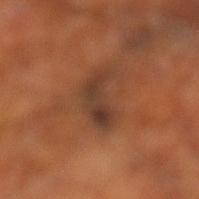workup — catalogued during a skin exam; not biopsied
image source — total-body-photography crop, ~15 mm field of view
automated metrics — an eccentricity of roughly 0.9 and two-axis asymmetry of about 0.5; an average lesion color of about L≈36 a*≈20 b*≈28 (CIELAB), roughly 8 lightness units darker than nearby skin, and a normalized lesion–skin contrast near 8; a nevus-likeness score of about 0/100 and lesion-presence confidence of about 85/100
location — the left lower leg
patient — male, about 70 years old